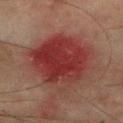Case summary:
– workup · imaged on a skin check; not biopsied
– lesion size · ≈8 mm
– site · the right forearm
– image source · 15 mm crop, total-body photography
– subject · male, aged 73–77
– lighting · cross-polarized